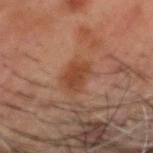Q: Was this lesion biopsied?
A: no biopsy performed (imaged during a skin exam)
Q: What is the lesion's diameter?
A: ~3 mm (longest diameter)
Q: Who is the patient?
A: male, aged approximately 50
Q: What lighting was used for the tile?
A: cross-polarized illumination
Q: What kind of image is this?
A: 15 mm crop, total-body photography
Q: Automated lesion metrics?
A: a lesion color around L≈32 a*≈19 b*≈25 in CIELAB and a normalized border contrast of about 7.5; a border-irregularity index near 2.5/10, a within-lesion color-variation index near 2/10, and a peripheral color-asymmetry measure near 0.5
Q: What is the anatomic site?
A: the head or neck